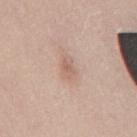Background:
On the back. A region of skin cropped from a whole-body photographic capture, roughly 15 mm wide. The total-body-photography lesion software estimated an outline eccentricity of about 0.7 (0 = round, 1 = elongated) and a symmetry-axis asymmetry near 0.25. The software also gave a lesion color around L≈62 a*≈19 b*≈27 in CIELAB, roughly 8 lightness units darker than nearby skin, and a lesion-to-skin contrast of about 5 (normalized; higher = more distinct). And it measured a nevus-likeness score of about 0/100 and a lesion-detection confidence of about 100/100. Captured under white-light illumination. Measured at roughly 2.5 mm in maximum diameter. A male patient, in their mid-40s.